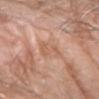Imaged during a routine full-body skin examination; the lesion was not biopsied and no histopathology is available. The lesion is located on the left forearm. The lesion-visualizer software estimated an average lesion color of about L≈60 a*≈22 b*≈31 (CIELAB) and roughly 7 lightness units darker than nearby skin. The software also gave a border-irregularity rating of about 6/10, a within-lesion color-variation index near 1.5/10, and a peripheral color-asymmetry measure near 0.5. The analysis additionally found lesion-presence confidence of about 95/100. The lesion's longest dimension is about 3 mm. A region of skin cropped from a whole-body photographic capture, roughly 15 mm wide. The subject is a male aged around 60.